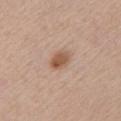Q: Is there a histopathology result?
A: total-body-photography surveillance lesion; no biopsy
Q: What kind of image is this?
A: ~15 mm crop, total-body skin-cancer survey
Q: Lesion location?
A: the left upper arm
Q: How large is the lesion?
A: about 3 mm
Q: Automated lesion metrics?
A: a mean CIELAB color near L≈56 a*≈19 b*≈30 and a normalized lesion–skin contrast near 8; lesion-presence confidence of about 100/100
Q: What lighting was used for the tile?
A: white-light
Q: Who is the patient?
A: male, about 60 years old